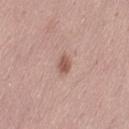Part of a total-body skin-imaging series; this lesion was reviewed on a skin check and was not flagged for biopsy.
The lesion is on the right thigh.
Cropped from a whole-body photographic skin survey; the tile spans about 15 mm.
The subject is a female aged around 25.
Imaged with white-light lighting.